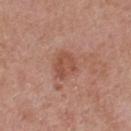This lesion was catalogued during total-body skin photography and was not selected for biopsy.